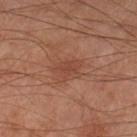follow-up: total-body-photography surveillance lesion; no biopsy
subject: male, about 70 years old
site: the left lower leg
image: total-body-photography crop, ~15 mm field of view
diameter: about 3 mm